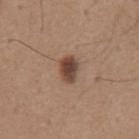Q: Was this lesion biopsied?
A: no biopsy performed (imaged during a skin exam)
Q: What are the patient's age and sex?
A: male, roughly 65 years of age
Q: What is the lesion's diameter?
A: about 3 mm
Q: Lesion location?
A: the abdomen
Q: How was this image acquired?
A: 15 mm crop, total-body photography
Q: Automated lesion metrics?
A: border irregularity of about 2 on a 0–10 scale and a within-lesion color-variation index near 4.5/10; an automated nevus-likeness rating near 95 out of 100 and a detector confidence of about 100 out of 100 that the crop contains a lesion